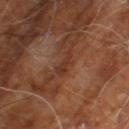Impression:
Imaged during a routine full-body skin examination; the lesion was not biopsied and no histopathology is available.
Image and clinical context:
Located on the leg. Measured at roughly 3 mm in maximum diameter. A male subject, about 60 years old. This is a cross-polarized tile. A roughly 15 mm field-of-view crop from a total-body skin photograph.